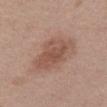notes: no biopsy performed (imaged during a skin exam)
site: the abdomen
tile lighting: white-light illumination
image: ~15 mm tile from a whole-body skin photo
automated metrics: a lesion color around L≈52 a*≈20 b*≈27 in CIELAB and roughly 9 lightness units darker than nearby skin; a border-irregularity index near 3.5/10, internal color variation of about 3.5 on a 0–10 scale, and radial color variation of about 1; a classifier nevus-likeness of about 40/100 and lesion-presence confidence of about 100/100
patient: female, aged 38–42
size: about 5.5 mm